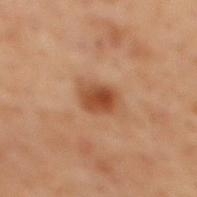biopsy status: imaged on a skin check; not biopsied
acquisition: 15 mm crop, total-body photography
size: about 3 mm
patient: male, about 70 years old
automated metrics: a border-irregularity index near 1.5/10, a color-variation rating of about 3.5/10, and a peripheral color-asymmetry measure near 1; a classifier nevus-likeness of about 95/100 and a detector confidence of about 100 out of 100 that the crop contains a lesion
location: the mid back
illumination: cross-polarized illumination